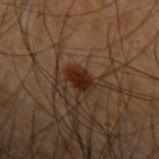This lesion was catalogued during total-body skin photography and was not selected for biopsy. The lesion is on the left forearm. A close-up tile cropped from a whole-body skin photograph, about 15 mm across. The lesion-visualizer software estimated a classifier nevus-likeness of about 85/100. Approximately 2.5 mm at its widest. A male patient, aged 48–52. Imaged with cross-polarized lighting.